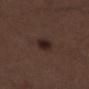follow-up: total-body-photography surveillance lesion; no biopsy | tile lighting: white-light | subject: male, about 50 years old | site: the leg | automated metrics: an area of roughly 5 mm², an outline eccentricity of about 0.6 (0 = round, 1 = elongated), and a symmetry-axis asymmetry near 0.15; a lesion color around L≈23 a*≈14 b*≈17 in CIELAB, roughly 8 lightness units darker than nearby skin, and a normalized lesion–skin contrast near 10 | imaging modality: ~15 mm tile from a whole-body skin photo | diameter: about 3 mm.